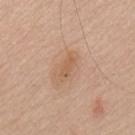<lesion>
<biopsy_status>not biopsied; imaged during a skin examination</biopsy_status>
<image>
  <source>total-body photography crop</source>
  <field_of_view_mm>15</field_of_view_mm>
</image>
<lesion_size>
  <long_diameter_mm_approx>2.5</long_diameter_mm_approx>
</lesion_size>
<lighting>white-light</lighting>
<site>upper back</site>
<patient>
  <sex>male</sex>
  <age_approx>65</age_approx>
</patient>
</lesion>